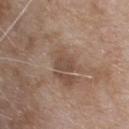The lesion was tiled from a total-body skin photograph and was not biopsied.
The lesion is on the upper back.
A female subject, in their mid-70s.
A 15 mm close-up extracted from a 3D total-body photography capture.
Longest diameter approximately 2.5 mm.
Captured under white-light illumination.
An algorithmic analysis of the crop reported a lesion area of about 3.5 mm², a shape eccentricity near 0.85, and two-axis asymmetry of about 0.15. And it measured border irregularity of about 1.5 on a 0–10 scale, a within-lesion color-variation index near 1.5/10, and radial color variation of about 0.5.